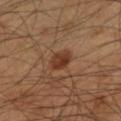The lesion was tiled from a total-body skin photograph and was not biopsied.
This image is a 15 mm lesion crop taken from a total-body photograph.
The patient is a male approximately 45 years of age.
Located on the arm.
An algorithmic analysis of the crop reported a lesion color around L≈34 a*≈21 b*≈29 in CIELAB, about 10 CIELAB-L* units darker than the surrounding skin, and a lesion-to-skin contrast of about 9 (normalized; higher = more distinct). The analysis additionally found radial color variation of about 1.5. The software also gave a detector confidence of about 100 out of 100 that the crop contains a lesion.